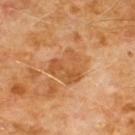notes: no biopsy performed (imaged during a skin exam)
imaging modality: 15 mm crop, total-body photography
location: the chest
patient: male, aged approximately 60
illumination: cross-polarized illumination
automated metrics: an area of roughly 12 mm² and a shape eccentricity near 0.45; a lesion color around L≈53 a*≈24 b*≈40 in CIELAB, roughly 8 lightness units darker than nearby skin, and a lesion-to-skin contrast of about 6.5 (normalized; higher = more distinct)
lesion size: about 4 mm